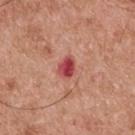Impression:
Recorded during total-body skin imaging; not selected for excision or biopsy.
Clinical summary:
Imaged with white-light lighting. The lesion-visualizer software estimated an area of roughly 5 mm², an eccentricity of roughly 0.7, and a symmetry-axis asymmetry near 0.2. And it measured a border-irregularity rating of about 2/10 and radial color variation of about 1.5. A 15 mm crop from a total-body photograph taken for skin-cancer surveillance. A male patient, aged around 55. The lesion is on the upper back.